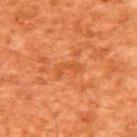Case summary:
• workup — no biopsy performed (imaged during a skin exam)
• anatomic site — the upper back
• subject — male, about 60 years old
• image-analysis metrics — a lesion color around L≈41 a*≈26 b*≈37 in CIELAB, roughly 5 lightness units darker than nearby skin, and a normalized lesion–skin contrast near 5
• lesion size — ≈3 mm
• acquisition — ~15 mm crop, total-body skin-cancer survey